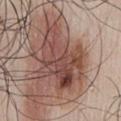Captured during whole-body skin photography for melanoma surveillance; the lesion was not biopsied. The recorded lesion diameter is about 8 mm. Captured under white-light illumination. A roughly 15 mm field-of-view crop from a total-body skin photograph. The lesion is on the chest. The total-body-photography lesion software estimated a border-irregularity index near 6/10. The patient is a male aged around 45.